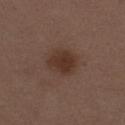Clinical impression: No biopsy was performed on this lesion — it was imaged during a full skin examination and was not determined to be concerning. Clinical summary: This image is a 15 mm lesion crop taken from a total-body photograph. Automated image analysis of the tile measured a shape-asymmetry score of about 0.15 (0 = symmetric). It also reported a lesion color around L≈33 a*≈17 b*≈24 in CIELAB, roughly 8 lightness units darker than nearby skin, and a lesion-to-skin contrast of about 8 (normalized; higher = more distinct). The analysis additionally found border irregularity of about 1.5 on a 0–10 scale, a color-variation rating of about 3/10, and radial color variation of about 1. A female subject about 40 years old. From the leg.